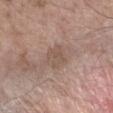The lesion is on the arm. A male subject aged 58–62. A 15 mm close-up extracted from a 3D total-body photography capture.